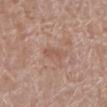Part of a total-body skin-imaging series; this lesion was reviewed on a skin check and was not flagged for biopsy.
This is a white-light tile.
This image is a 15 mm lesion crop taken from a total-body photograph.
Approximately 3 mm at its widest.
An algorithmic analysis of the crop reported a shape-asymmetry score of about 0.45 (0 = symmetric).
A male subject, roughly 70 years of age.
Located on the left lower leg.